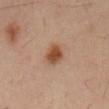| feature | finding |
|---|---|
| biopsy status | total-body-photography surveillance lesion; no biopsy |